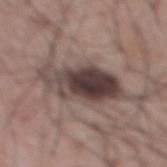biopsy_status: not biopsied; imaged during a skin examination
site: mid back
image:
  source: total-body photography crop
  field_of_view_mm: 15
patient:
  sex: male
  age_approx: 70
lighting: white-light